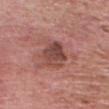Impression: The lesion was tiled from a total-body skin photograph and was not biopsied. Background: The patient is a male roughly 70 years of age. A roughly 15 mm field-of-view crop from a total-body skin photograph. The lesion is on the head or neck.